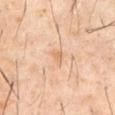Captured during whole-body skin photography for melanoma surveillance; the lesion was not biopsied. About 2.5 mm across. Located on the abdomen. A 15 mm close-up extracted from a 3D total-body photography capture. A male subject, roughly 60 years of age. The tile uses cross-polarized illumination.